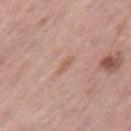This lesion was catalogued during total-body skin photography and was not selected for biopsy.
On the leg.
A female subject, aged 58 to 62.
A 15 mm close-up tile from a total-body photography series done for melanoma screening.